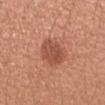Findings:
• workup — catalogued during a skin exam; not biopsied
• tile lighting — white-light
• lesion diameter — about 4.5 mm
• image — ~15 mm tile from a whole-body skin photo
• body site — the left upper arm
• automated metrics — a lesion color around L≈51 a*≈26 b*≈31 in CIELAB, roughly 10 lightness units darker than nearby skin, and a lesion-to-skin contrast of about 7 (normalized; higher = more distinct); a border-irregularity rating of about 2/10 and a peripheral color-asymmetry measure near 1
• subject — female, aged around 40